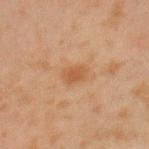A close-up tile cropped from a whole-body skin photograph, about 15 mm across.
The lesion's longest dimension is about 2.5 mm.
An algorithmic analysis of the crop reported an area of roughly 4 mm², a shape eccentricity near 0.7, and two-axis asymmetry of about 0.25. And it measured a border-irregularity index near 2/10, a within-lesion color-variation index near 1/10, and peripheral color asymmetry of about 0.5. The analysis additionally found an automated nevus-likeness rating near 20 out of 100 and a lesion-detection confidence of about 100/100.
A male subject aged approximately 45.
The lesion is on the arm.
The tile uses cross-polarized illumination.